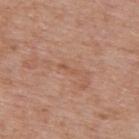Impression: This lesion was catalogued during total-body skin photography and was not selected for biopsy. Image and clinical context: A male subject, approximately 75 years of age. An algorithmic analysis of the crop reported an average lesion color of about L≈54 a*≈22 b*≈32 (CIELAB), about 6 CIELAB-L* units darker than the surrounding skin, and a normalized lesion–skin contrast near 4.5. Located on the upper back. Measured at roughly 2.5 mm in maximum diameter. Captured under white-light illumination. Cropped from a whole-body photographic skin survey; the tile spans about 15 mm.